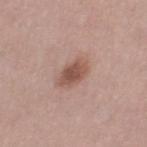Impression: This lesion was catalogued during total-body skin photography and was not selected for biopsy. Clinical summary: Located on the right thigh. A 15 mm crop from a total-body photograph taken for skin-cancer surveillance. Imaged with white-light lighting. The patient is a female about 40 years old. An algorithmic analysis of the crop reported a shape-asymmetry score of about 0.3 (0 = symmetric). The analysis additionally found an average lesion color of about L≈51 a*≈21 b*≈25 (CIELAB), a lesion–skin lightness drop of about 12, and a normalized border contrast of about 8.5.